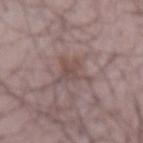No biopsy was performed on this lesion — it was imaged during a full skin examination and was not determined to be concerning. Approximately 4 mm at its widest. Automated tile analysis of the lesion measured an automated nevus-likeness rating near 0 out of 100 and a lesion-detection confidence of about 90/100. A 15 mm crop from a total-body photograph taken for skin-cancer surveillance. A male subject, aged 48–52. The lesion is on the left forearm.